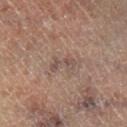biopsy_status: not biopsied; imaged during a skin examination
site: right lower leg
image:
  source: total-body photography crop
  field_of_view_mm: 15
patient:
  sex: male
  age_approx: 65